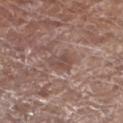* image source · total-body-photography crop, ~15 mm field of view
* patient · female, aged 78 to 82
* anatomic site · the left lower leg